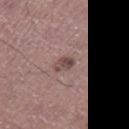This lesion was catalogued during total-body skin photography and was not selected for biopsy.
The lesion is on the right thigh.
The tile uses white-light illumination.
A male subject, aged 43 to 47.
A 15 mm close-up tile from a total-body photography series done for melanoma screening.
The lesion-visualizer software estimated an area of roughly 3.5 mm², a shape eccentricity near 0.75, and two-axis asymmetry of about 0.2.